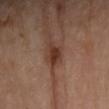This lesion was catalogued during total-body skin photography and was not selected for biopsy.
The patient is a female aged 63–67.
Located on the left forearm.
This image is a 15 mm lesion crop taken from a total-body photograph.